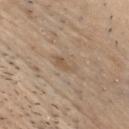The lesion was tiled from a total-body skin photograph and was not biopsied.
From the head or neck.
Cropped from a total-body skin-imaging series; the visible field is about 15 mm.
A male patient aged 33 to 37.